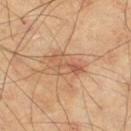Clinical impression: Part of a total-body skin-imaging series; this lesion was reviewed on a skin check and was not flagged for biopsy. Image and clinical context: A 15 mm crop from a total-body photograph taken for skin-cancer surveillance. The lesion is on the right thigh. The subject is a male aged 68 to 72. The tile uses cross-polarized illumination.